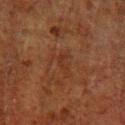Q: What kind of image is this?
A: ~15 mm crop, total-body skin-cancer survey
Q: Patient demographics?
A: male, aged 78 to 82
Q: What lighting was used for the tile?
A: cross-polarized illumination
Q: Lesion size?
A: ~3.5 mm (longest diameter)
Q: What is the anatomic site?
A: the left lower leg